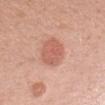Recorded during total-body skin imaging; not selected for excision or biopsy. The tile uses white-light illumination. A 15 mm crop from a total-body photograph taken for skin-cancer surveillance. The patient is a female aged around 40. From the left upper arm. Approximately 4 mm at its widest.